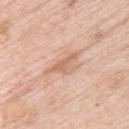biopsy_status: not biopsied; imaged during a skin examination
patient:
  sex: female
  age_approx: 65
image:
  source: total-body photography crop
  field_of_view_mm: 15
automated_metrics:
  nevus_likeness_0_100: 0
site: upper back
lighting: white-light
lesion_size:
  long_diameter_mm_approx: 4.5